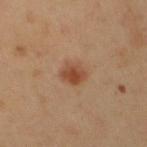<record>
<biopsy_status>not biopsied; imaged during a skin examination</biopsy_status>
<image>
  <source>total-body photography crop</source>
  <field_of_view_mm>15</field_of_view_mm>
</image>
<site>chest</site>
<patient>
  <sex>male</sex>
  <age_approx>50</age_approx>
</patient>
</record>